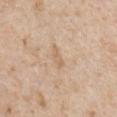The lesion was photographed on a routine skin check and not biopsied; there is no pathology result. The subject is a male aged 63–67. Cropped from a whole-body photographic skin survey; the tile spans about 15 mm. On the right upper arm. Automated image analysis of the tile measured a shape eccentricity near 0.85 and two-axis asymmetry of about 0.2. It also reported an automated nevus-likeness rating near 0 out of 100 and lesion-presence confidence of about 100/100.